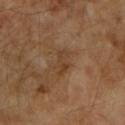biopsy_status: not biopsied; imaged during a skin examination
lesion_size:
  long_diameter_mm_approx: 3.0
lighting: cross-polarized
patient:
  sex: female
  age_approx: 70
image:
  source: total-body photography crop
  field_of_view_mm: 15
site: left forearm